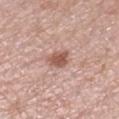Part of a total-body skin-imaging series; this lesion was reviewed on a skin check and was not flagged for biopsy.
Approximately 2.5 mm at its widest.
A region of skin cropped from a whole-body photographic capture, roughly 15 mm wide.
A female subject in their mid- to late 50s.
Automated image analysis of the tile measured peripheral color asymmetry of about 0.5. It also reported an automated nevus-likeness rating near 85 out of 100 and lesion-presence confidence of about 100/100.
The lesion is on the right lower leg.